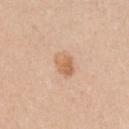Imaged during a routine full-body skin examination; the lesion was not biopsied and no histopathology is available.
A 15 mm close-up extracted from a 3D total-body photography capture.
A female patient, aged 43 to 47.
Approximately 3 mm at its widest.
From the front of the torso.